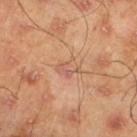workup — total-body-photography surveillance lesion; no biopsy
anatomic site — the left lower leg
patient — male, roughly 45 years of age
image source — 15 mm crop, total-body photography